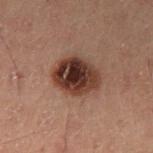Findings:
– workup · no biopsy performed (imaged during a skin exam)
– lighting · cross-polarized
– subject · male, in their 60s
– anatomic site · the left thigh
– lesion diameter · about 5 mm
– acquisition · 15 mm crop, total-body photography